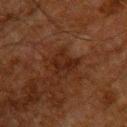This lesion was catalogued during total-body skin photography and was not selected for biopsy. Longest diameter approximately 3.5 mm. A male subject approximately 60 years of age. On the upper back. A 15 mm close-up tile from a total-body photography series done for melanoma screening.